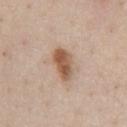automated_metrics:
  cielab_L: 56
  cielab_a: 19
  cielab_b: 31
  vs_skin_darker_L: 13.0
  vs_skin_contrast_norm: 9.5
  border_irregularity_0_10: 2.5
  color_variation_0_10: 4.5
site: chest
lesion_size:
  long_diameter_mm_approx: 4.0
patient:
  sex: male
  age_approx: 60
image:
  source: total-body photography crop
  field_of_view_mm: 15
lighting: white-light